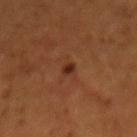Q: Is there a histopathology result?
A: no biopsy performed (imaged during a skin exam)
Q: Lesion location?
A: the back
Q: Patient demographics?
A: male, aged around 45
Q: What is the imaging modality?
A: 15 mm crop, total-body photography